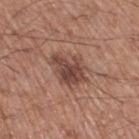Q: Is there a histopathology result?
A: no biopsy performed (imaged during a skin exam)
Q: How was the tile lit?
A: white-light illumination
Q: Where on the body is the lesion?
A: the right thigh
Q: What did automated image analysis measure?
A: an area of roughly 10 mm² and a shape eccentricity near 0.7
Q: What kind of image is this?
A: ~15 mm crop, total-body skin-cancer survey
Q: How large is the lesion?
A: ~4.5 mm (longest diameter)
Q: Patient demographics?
A: male, aged around 65